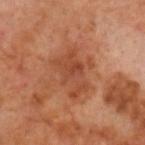The lesion was photographed on a routine skin check and not biopsied; there is no pathology result.
This is a cross-polarized tile.
On the head or neck.
Approximately 5 mm at its widest.
A male patient roughly 60 years of age.
An algorithmic analysis of the crop reported a shape eccentricity near 0.85 and a symmetry-axis asymmetry near 0.5.
This image is a 15 mm lesion crop taken from a total-body photograph.